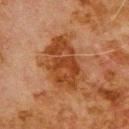Case summary:
• biopsy status: catalogued during a skin exam; not biopsied
• illumination: cross-polarized
• location: the upper back
• lesion size: ~7 mm (longest diameter)
• image: ~15 mm crop, total-body skin-cancer survey
• patient: male, about 80 years old